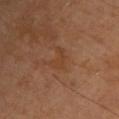Assessment:
Imaged during a routine full-body skin examination; the lesion was not biopsied and no histopathology is available.
Clinical summary:
A male subject roughly 70 years of age. Located on the upper back. Cropped from a whole-body photographic skin survey; the tile spans about 15 mm.